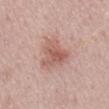No biopsy was performed on this lesion — it was imaged during a full skin examination and was not determined to be concerning. A male subject, approximately 70 years of age. A 15 mm close-up extracted from a 3D total-body photography capture. From the mid back. The recorded lesion diameter is about 4.5 mm. Captured under white-light illumination.